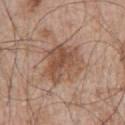Findings:
* follow-up · imaged on a skin check; not biopsied
* image source · total-body-photography crop, ~15 mm field of view
* TBP lesion metrics · a lesion area of about 19 mm² and an eccentricity of roughly 0.35; roughly 9 lightness units darker than nearby skin and a normalized lesion–skin contrast near 7; a classifier nevus-likeness of about 15/100 and lesion-presence confidence of about 100/100
* patient · male, aged 63 to 67
* body site · the left upper arm